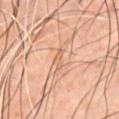follow-up: no biopsy performed (imaged during a skin exam)
subject: male, aged 43–47
image-analysis metrics: a lesion area of about 2.5 mm², an outline eccentricity of about 0.95 (0 = round, 1 = elongated), and a symmetry-axis asymmetry near 0.4; a classifier nevus-likeness of about 0/100 and a detector confidence of about 55 out of 100 that the crop contains a lesion
lighting: cross-polarized illumination
image: ~15 mm tile from a whole-body skin photo
body site: the chest